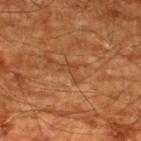{
  "biopsy_status": "not biopsied; imaged during a skin examination",
  "patient": {
    "sex": "male",
    "age_approx": 60
  },
  "lighting": "cross-polarized",
  "site": "upper back",
  "image": {
    "source": "total-body photography crop",
    "field_of_view_mm": 15
  }
}